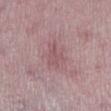biopsy status: no biopsy performed (imaged during a skin exam) | subject: female, about 50 years old | automated lesion analysis: a lesion area of about 6.5 mm², an outline eccentricity of about 0.75 (0 = round, 1 = elongated), and a symmetry-axis asymmetry near 0.35; an average lesion color of about L≈54 a*≈22 b*≈16 (CIELAB), a lesion–skin lightness drop of about 7, and a normalized border contrast of about 4.5; a nevus-likeness score of about 0/100 and a detector confidence of about 75 out of 100 that the crop contains a lesion | size: about 4 mm | anatomic site: the left lower leg | tile lighting: white-light | image: 15 mm crop, total-body photography.